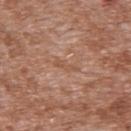  biopsy_status: not biopsied; imaged during a skin examination
  image:
    source: total-body photography crop
    field_of_view_mm: 15
  site: upper back
  patient:
    sex: male
    age_approx: 45